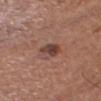Clinical impression:
Captured during whole-body skin photography for melanoma surveillance; the lesion was not biopsied.
Context:
Cropped from a total-body skin-imaging series; the visible field is about 15 mm. A male subject, approximately 55 years of age. The recorded lesion diameter is about 3 mm. This is a white-light tile. On the head or neck.